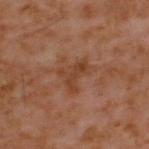Assessment:
Imaged during a routine full-body skin examination; the lesion was not biopsied and no histopathology is available.
Clinical summary:
Measured at roughly 4 mm in maximum diameter. From the upper back. The subject is a male aged approximately 60. This is a cross-polarized tile. A lesion tile, about 15 mm wide, cut from a 3D total-body photograph.